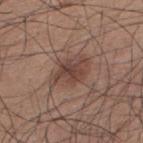Impression:
The lesion was tiled from a total-body skin photograph and was not biopsied.
Clinical summary:
Approximately 5 mm at its widest. This is a white-light tile. A close-up tile cropped from a whole-body skin photograph, about 15 mm across. The patient is a male aged 33–37. Automated tile analysis of the lesion measured a within-lesion color-variation index near 4/10. On the upper back.